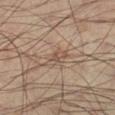Clinical impression: The lesion was photographed on a routine skin check and not biopsied; there is no pathology result. Clinical summary: From the left lower leg. A region of skin cropped from a whole-body photographic capture, roughly 15 mm wide. A male patient aged 38–42. The lesion-visualizer software estimated a lesion area of about 4.5 mm², a shape eccentricity near 0.85, and two-axis asymmetry of about 0.35. And it measured an average lesion color of about L≈50 a*≈15 b*≈25 (CIELAB), roughly 8 lightness units darker than nearby skin, and a lesion-to-skin contrast of about 5.5 (normalized; higher = more distinct). The software also gave a border-irregularity index near 4/10, internal color variation of about 3 on a 0–10 scale, and a peripheral color-asymmetry measure near 0.5. The recorded lesion diameter is about 3.5 mm.